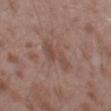This lesion was catalogued during total-body skin photography and was not selected for biopsy. Captured under white-light illumination. A male patient, roughly 45 years of age. Located on the left thigh. This image is a 15 mm lesion crop taken from a total-body photograph. The lesion's longest dimension is about 5.5 mm.